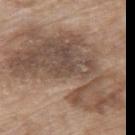Acquisition and patient details:
Located on the back. The tile uses white-light illumination. Cropped from a total-body skin-imaging series; the visible field is about 15 mm. Automated image analysis of the tile measured a footprint of about 75 mm², an eccentricity of roughly 0.85, and two-axis asymmetry of about 0.4. It also reported a mean CIELAB color near L≈50 a*≈15 b*≈26 and roughly 11 lightness units darker than nearby skin. It also reported a border-irregularity index near 7.5/10, a color-variation rating of about 6.5/10, and peripheral color asymmetry of about 2. Measured at roughly 14.5 mm in maximum diameter. A female subject about 75 years old.